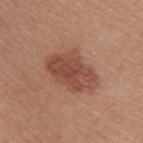Clinical impression:
The lesion was photographed on a routine skin check and not biopsied; there is no pathology result.
Acquisition and patient details:
This is a white-light tile. The total-body-photography lesion software estimated a nevus-likeness score of about 80/100 and a detector confidence of about 100 out of 100 that the crop contains a lesion. The recorded lesion diameter is about 6 mm. On the back. A 15 mm close-up extracted from a 3D total-body photography capture. A male subject, aged 38 to 42.